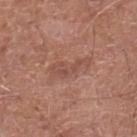Assessment: Imaged during a routine full-body skin examination; the lesion was not biopsied and no histopathology is available. Acquisition and patient details: The lesion is on the left lower leg. The lesion-visualizer software estimated a lesion area of about 5.5 mm², an eccentricity of roughly 0.95, and a shape-asymmetry score of about 0.5 (0 = symmetric). Longest diameter approximately 4.5 mm. The subject is a male approximately 65 years of age. Captured under white-light illumination. Cropped from a total-body skin-imaging series; the visible field is about 15 mm.